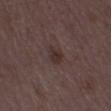Findings:
• workup · no biopsy performed (imaged during a skin exam)
• TBP lesion metrics · about 7 CIELAB-L* units darker than the surrounding skin and a lesion-to-skin contrast of about 7 (normalized; higher = more distinct); internal color variation of about 1.5 on a 0–10 scale
• subject · female, roughly 35 years of age
• lesion size · about 3 mm
• imaging modality · 15 mm crop, total-body photography
• site · the right thigh
• tile lighting · white-light